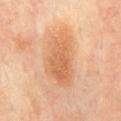Findings:
- follow-up · catalogued during a skin exam; not biopsied
- location · the back
- lesion diameter · ~7 mm (longest diameter)
- lighting · cross-polarized
- subject · female, aged around 60
- image source · total-body-photography crop, ~15 mm field of view